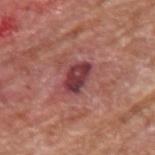Assessment: Imaged during a routine full-body skin examination; the lesion was not biopsied and no histopathology is available. Context: Cropped from a total-body skin-imaging series; the visible field is about 15 mm. The subject is a male approximately 65 years of age. Captured under white-light illumination. About 3.5 mm across. The lesion is on the upper back.